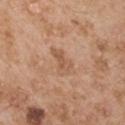Q: Was this lesion biopsied?
A: total-body-photography surveillance lesion; no biopsy
Q: Where on the body is the lesion?
A: the left upper arm
Q: How was this image acquired?
A: 15 mm crop, total-body photography
Q: What did automated image analysis measure?
A: a border-irregularity index near 4/10, a color-variation rating of about 3/10, and a peripheral color-asymmetry measure near 1
Q: How was the tile lit?
A: white-light
Q: What is the lesion's diameter?
A: ~3.5 mm (longest diameter)
Q: Who is the patient?
A: male, aged 53–57